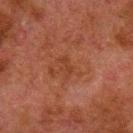Q: Was this lesion biopsied?
A: catalogued during a skin exam; not biopsied
Q: How was this image acquired?
A: total-body-photography crop, ~15 mm field of view
Q: Automated lesion metrics?
A: a mean CIELAB color near L≈31 a*≈21 b*≈28, a lesion–skin lightness drop of about 4, and a lesion-to-skin contrast of about 5 (normalized; higher = more distinct); a border-irregularity rating of about 4.5/10, a within-lesion color-variation index near 0/10, and radial color variation of about 0
Q: Lesion location?
A: the left lower leg
Q: What lighting was used for the tile?
A: cross-polarized
Q: Lesion size?
A: ≈2.5 mm
Q: Patient demographics?
A: male, in their 80s